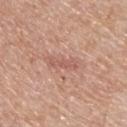  lighting: white-light
  lesion_size:
    long_diameter_mm_approx: 3.0
  automated_metrics:
    cielab_L: 57
    cielab_a: 24
    cielab_b: 27
    vs_skin_contrast_norm: 5.0
    border_irregularity_0_10: 5.0
    peripheral_color_asymmetry: 0.0
    nevus_likeness_0_100: 0
  image:
    source: total-body photography crop
    field_of_view_mm: 15
  site: upper back
  patient:
    sex: male
    age_approx: 55